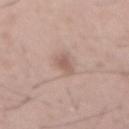Part of a total-body skin-imaging series; this lesion was reviewed on a skin check and was not flagged for biopsy.
A roughly 15 mm field-of-view crop from a total-body skin photograph.
From the mid back.
Imaged with white-light lighting.
Approximately 3 mm at its widest.
The subject is a male in their mid-50s.